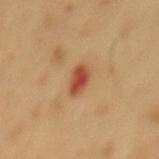Part of a total-body skin-imaging series; this lesion was reviewed on a skin check and was not flagged for biopsy.
The lesion is located on the mid back.
A 15 mm crop from a total-body photograph taken for skin-cancer surveillance.
Captured under cross-polarized illumination.
The subject is a male aged 48–52.